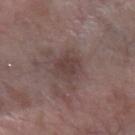Imaged during a routine full-body skin examination; the lesion was not biopsied and no histopathology is available. A female subject aged 63–67. This is a white-light tile. The lesion is on the arm. Cropped from a total-body skin-imaging series; the visible field is about 15 mm. Approximately 4 mm at its widest. The lesion-visualizer software estimated a footprint of about 9.5 mm², an eccentricity of roughly 0.65, and a shape-asymmetry score of about 0.2 (0 = symmetric). The software also gave a mean CIELAB color near L≈41 a*≈15 b*≈18, a lesion–skin lightness drop of about 7, and a normalized lesion–skin contrast near 6.5. The software also gave a peripheral color-asymmetry measure near 0.5. The analysis additionally found an automated nevus-likeness rating near 0 out of 100 and lesion-presence confidence of about 100/100.